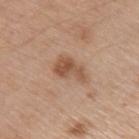Findings:
• workup · no biopsy performed (imaged during a skin exam)
• image · ~15 mm tile from a whole-body skin photo
• subject · male, about 60 years old
• location · the left upper arm